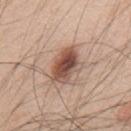follow-up: no biopsy performed (imaged during a skin exam)
image-analysis metrics: a footprint of about 11 mm² and a symmetry-axis asymmetry near 0.25; border irregularity of about 2.5 on a 0–10 scale, internal color variation of about 7.5 on a 0–10 scale, and radial color variation of about 2
subject: male, aged 48–52
acquisition: ~15 mm tile from a whole-body skin photo
lighting: white-light
body site: the back